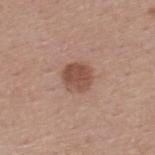The lesion was photographed on a routine skin check and not biopsied; there is no pathology result. Located on the upper back. Measured at roughly 3 mm in maximum diameter. A male subject aged 38 to 42. A 15 mm close-up extracted from a 3D total-body photography capture.